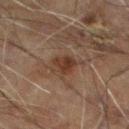Impression: The lesion was photographed on a routine skin check and not biopsied; there is no pathology result. Clinical summary: Automated image analysis of the tile measured a mean CIELAB color near L≈26 a*≈15 b*≈22, about 7 CIELAB-L* units darker than the surrounding skin, and a lesion-to-skin contrast of about 8 (normalized; higher = more distinct). The software also gave a border-irregularity index near 3/10, internal color variation of about 2.5 on a 0–10 scale, and peripheral color asymmetry of about 1. It also reported an automated nevus-likeness rating near 25 out of 100 and a lesion-detection confidence of about 100/100. Measured at roughly 2.5 mm in maximum diameter. The subject is a male approximately 60 years of age. From the left lower leg. Imaged with cross-polarized lighting. A 15 mm crop from a total-body photograph taken for skin-cancer surveillance.